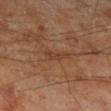Assessment:
Imaged during a routine full-body skin examination; the lesion was not biopsied and no histopathology is available.
Acquisition and patient details:
The lesion's longest dimension is about 3 mm. A 15 mm crop from a total-body photograph taken for skin-cancer surveillance. A male subject, aged around 70. On the right lower leg. The lesion-visualizer software estimated a footprint of about 3.5 mm². The software also gave a mean CIELAB color near L≈39 a*≈20 b*≈29, about 6 CIELAB-L* units darker than the surrounding skin, and a normalized border contrast of about 5. The analysis additionally found a within-lesion color-variation index near 1/10 and radial color variation of about 0. It also reported a classifier nevus-likeness of about 0/100.